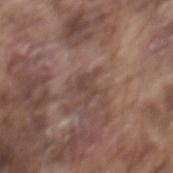Acquisition and patient details: From the back. A roughly 15 mm field-of-view crop from a total-body skin photograph. A male subject, aged 73–77. Imaged with white-light lighting. Longest diameter approximately 3 mm.